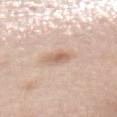<record>
  <biopsy_status>not biopsied; imaged during a skin examination</biopsy_status>
  <image>
    <source>total-body photography crop</source>
    <field_of_view_mm>15</field_of_view_mm>
  </image>
  <automated_metrics>
    <eccentricity>0.7</eccentricity>
    <cielab_L>64</cielab_L>
    <cielab_a>18</cielab_a>
    <cielab_b>28</cielab_b>
    <vs_skin_darker_L>10.0</vs_skin_darker_L>
    <border_irregularity_0_10>2.0</border_irregularity_0_10>
    <color_variation_0_10>4.5</color_variation_0_10>
    <nevus_likeness_0_100>35</nevus_likeness_0_100>
    <lesion_detection_confidence_0_100>100</lesion_detection_confidence_0_100>
  </automated_metrics>
  <lighting>white-light</lighting>
  <site>mid back</site>
  <lesion_size>
    <long_diameter_mm_approx>2.5</long_diameter_mm_approx>
  </lesion_size>
  <patient>
    <sex>female</sex>
    <age_approx>65</age_approx>
  </patient>
</record>